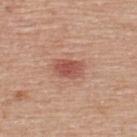Part of a total-body skin-imaging series; this lesion was reviewed on a skin check and was not flagged for biopsy.
A male patient about 55 years old.
Located on the upper back.
A 15 mm close-up extracted from a 3D total-body photography capture.
Approximately 3.5 mm at its widest.
Imaged with white-light lighting.